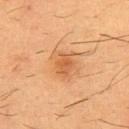notes = total-body-photography surveillance lesion; no biopsy
body site = the chest
patient = male, aged 53 to 57
lesion size = about 2.5 mm
imaging modality = ~15 mm tile from a whole-body skin photo
illumination = cross-polarized